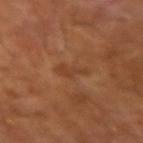No biopsy was performed on this lesion — it was imaged during a full skin examination and was not determined to be concerning.
Automated image analysis of the tile measured a footprint of about 4 mm², an eccentricity of roughly 0.85, and a shape-asymmetry score of about 0.35 (0 = symmetric). It also reported a normalized border contrast of about 5. It also reported a border-irregularity index near 4.5/10, a color-variation rating of about 1/10, and a peripheral color-asymmetry measure near 0. The analysis additionally found a nevus-likeness score of about 0/100 and lesion-presence confidence of about 100/100.
A male patient approximately 65 years of age.
A roughly 15 mm field-of-view crop from a total-body skin photograph.